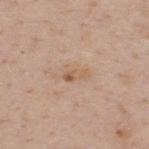Recorded during total-body skin imaging; not selected for excision or biopsy.
The patient is a male approximately 40 years of age.
On the upper back.
A 15 mm close-up tile from a total-body photography series done for melanoma screening.